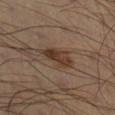Clinical impression:
Imaged during a routine full-body skin examination; the lesion was not biopsied and no histopathology is available.
Background:
The subject is a male approximately 65 years of age. Located on the right lower leg. Cropped from a whole-body photographic skin survey; the tile spans about 15 mm. Measured at roughly 4.5 mm in maximum diameter.